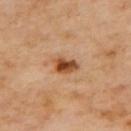Q: Was a biopsy performed?
A: catalogued during a skin exam; not biopsied
Q: Lesion size?
A: ≈3 mm
Q: How was the tile lit?
A: cross-polarized illumination
Q: What is the imaging modality?
A: ~15 mm tile from a whole-body skin photo
Q: Automated lesion metrics?
A: a lesion color around L≈50 a*≈25 b*≈38 in CIELAB, roughly 16 lightness units darker than nearby skin, and a normalized border contrast of about 11
Q: Lesion location?
A: the upper back